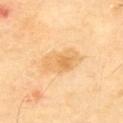Findings:
– illumination — cross-polarized
– lesion size — ≈5.5 mm
– subject — male, approximately 65 years of age
– image — 15 mm crop, total-body photography
– site — the upper back